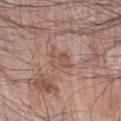{"biopsy_status": "not biopsied; imaged during a skin examination", "lighting": "white-light", "site": "left forearm", "patient": {"sex": "male", "age_approx": 70}, "image": {"source": "total-body photography crop", "field_of_view_mm": 15}, "automated_metrics": {"nevus_likeness_0_100": 0, "lesion_detection_confidence_0_100": 100}}